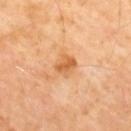workup: total-body-photography surveillance lesion; no biopsy
lesion size: ≈2.5 mm
subject: male, approximately 55 years of age
image: total-body-photography crop, ~15 mm field of view
TBP lesion metrics: a border-irregularity rating of about 2.5/10 and a color-variation rating of about 3/10; a classifier nevus-likeness of about 5/100 and a detector confidence of about 100 out of 100 that the crop contains a lesion
body site: the mid back